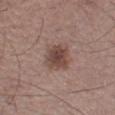Impression: Captured during whole-body skin photography for melanoma surveillance; the lesion was not biopsied. Clinical summary: A male subject about 50 years old. A 15 mm close-up extracted from a 3D total-body photography capture. On the left lower leg. Automated image analysis of the tile measured an eccentricity of roughly 0.6 and a shape-asymmetry score of about 0.2 (0 = symmetric). And it measured an average lesion color of about L≈46 a*≈18 b*≈23 (CIELAB), a lesion–skin lightness drop of about 11, and a normalized border contrast of about 8. The software also gave a lesion-detection confidence of about 100/100. Longest diameter approximately 4 mm. Captured under white-light illumination.